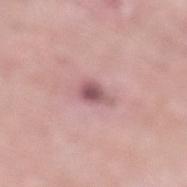Q: Is there a histopathology result?
A: imaged on a skin check; not biopsied
Q: How was the tile lit?
A: white-light illumination
Q: Lesion size?
A: about 3 mm
Q: What is the anatomic site?
A: the left lower leg
Q: How was this image acquired?
A: ~15 mm tile from a whole-body skin photo
Q: Who is the patient?
A: male, aged around 85 or older